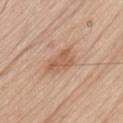<tbp_lesion>
  <biopsy_status>not biopsied; imaged during a skin examination</biopsy_status>
  <patient>
    <sex>male</sex>
    <age_approx>80</age_approx>
  </patient>
  <lighting>white-light</lighting>
  <site>back</site>
  <image>
    <source>total-body photography crop</source>
    <field_of_view_mm>15</field_of_view_mm>
  </image>
</tbp_lesion>